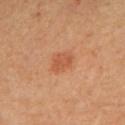This lesion was catalogued during total-body skin photography and was not selected for biopsy.
Imaged with cross-polarized lighting.
A female subject, aged approximately 30.
The lesion-visualizer software estimated a lesion area of about 4 mm², an outline eccentricity of about 0.7 (0 = round, 1 = elongated), and two-axis asymmetry of about 0.35. The analysis additionally found an automated nevus-likeness rating near 45 out of 100 and lesion-presence confidence of about 100/100.
Approximately 2.5 mm at its widest.
Cropped from a whole-body photographic skin survey; the tile spans about 15 mm.
The lesion is on the upper back.